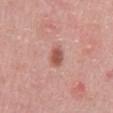Clinical impression:
Imaged during a routine full-body skin examination; the lesion was not biopsied and no histopathology is available.
Clinical summary:
The subject is a male approximately 50 years of age. The lesion is on the abdomen. A 15 mm close-up tile from a total-body photography series done for melanoma screening.